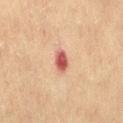No biopsy was performed on this lesion — it was imaged during a full skin examination and was not determined to be concerning.
A female subject aged around 55.
This is a cross-polarized tile.
Cropped from a whole-body photographic skin survey; the tile spans about 15 mm.
The lesion-visualizer software estimated an eccentricity of roughly 0.7 and two-axis asymmetry of about 0.2. It also reported a normalized border contrast of about 10. It also reported a classifier nevus-likeness of about 0/100 and lesion-presence confidence of about 100/100.
Approximately 2.5 mm at its widest.
Located on the right thigh.